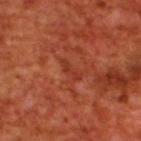biopsy status: no biopsy performed (imaged during a skin exam); subject: male, aged around 70; body site: the upper back; tile lighting: cross-polarized illumination; image: 15 mm crop, total-body photography; lesion diameter: ~3 mm (longest diameter).